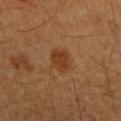notes = imaged on a skin check; not biopsied | site = the right forearm | illumination = cross-polarized illumination | imaging modality = 15 mm crop, total-body photography | patient = female, approximately 40 years of age.